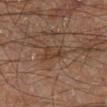Assessment: The lesion was tiled from a total-body skin photograph and was not biopsied. Acquisition and patient details: A male patient approximately 65 years of age. Located on the right lower leg. Cropped from a total-body skin-imaging series; the visible field is about 15 mm.